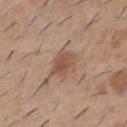  biopsy_status: not biopsied; imaged during a skin examination
  image:
    source: total-body photography crop
    field_of_view_mm: 15
  site: chest
  patient:
    sex: male
    age_approx: 40